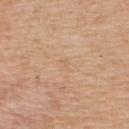workup — no biopsy performed (imaged during a skin exam) | patient — female, aged 43–47 | site — the upper back | image-analysis metrics — a lesion area of about 1.5 mm² and an outline eccentricity of about 0.7 (0 = round, 1 = elongated); a border-irregularity rating of about 3.5/10, internal color variation of about 0 on a 0–10 scale, and peripheral color asymmetry of about 0; an automated nevus-likeness rating near 0 out of 100 and a lesion-detection confidence of about 100/100 | acquisition — total-body-photography crop, ~15 mm field of view | diameter — about 1.5 mm.